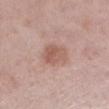Clinical impression: Part of a total-body skin-imaging series; this lesion was reviewed on a skin check and was not flagged for biopsy. Context: Imaged with white-light lighting. The subject is a female aged 48 to 52. A lesion tile, about 15 mm wide, cut from a 3D total-body photograph. Located on the left lower leg. An algorithmic analysis of the crop reported a lesion area of about 8.5 mm², an eccentricity of roughly 0.65, and a shape-asymmetry score of about 0.2 (0 = symmetric). The analysis additionally found a mean CIELAB color near L≈57 a*≈21 b*≈26 and roughly 9 lightness units darker than nearby skin.